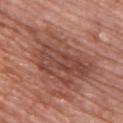follow-up = imaged on a skin check; not biopsied
subject = male, aged 48–52
image = ~15 mm tile from a whole-body skin photo
lesion size = ≈9.5 mm
illumination = white-light
anatomic site = the upper back